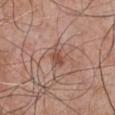Case summary:
• workup — total-body-photography surveillance lesion; no biopsy
• subject — male, approximately 70 years of age
• anatomic site — the chest
• imaging modality — ~15 mm crop, total-body skin-cancer survey